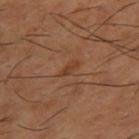Recorded during total-body skin imaging; not selected for excision or biopsy. Located on the right thigh. Longest diameter approximately 2.5 mm. Imaged with cross-polarized lighting. A male patient aged around 65. A 15 mm close-up extracted from a 3D total-body photography capture.